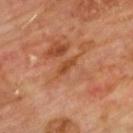Assessment: No biopsy was performed on this lesion — it was imaged during a full skin examination and was not determined to be concerning. Acquisition and patient details: Approximately 2.5 mm at its widest. The lesion is located on the upper back. Captured under cross-polarized illumination. Automated tile analysis of the lesion measured an average lesion color of about L≈45 a*≈26 b*≈37 (CIELAB), about 8 CIELAB-L* units darker than the surrounding skin, and a lesion-to-skin contrast of about 7 (normalized; higher = more distinct). The analysis additionally found a classifier nevus-likeness of about 0/100. Cropped from a total-body skin-imaging series; the visible field is about 15 mm. A male patient approximately 60 years of age.